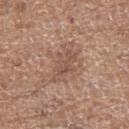Image and clinical context: Located on the left thigh. A female patient roughly 75 years of age. This is a white-light tile. A 15 mm crop from a total-body photograph taken for skin-cancer surveillance.